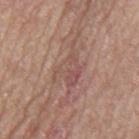Clinical impression: Captured during whole-body skin photography for melanoma surveillance; the lesion was not biopsied. Image and clinical context: The total-body-photography lesion software estimated an average lesion color of about L≈51 a*≈20 b*≈23 (CIELAB) and a lesion-to-skin contrast of about 5.5 (normalized; higher = more distinct). A male patient in their mid-60s. Longest diameter approximately 5 mm. The lesion is located on the mid back. Imaged with white-light lighting. A 15 mm close-up extracted from a 3D total-body photography capture.